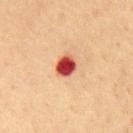Clinical summary: From the chest. A lesion tile, about 15 mm wide, cut from a 3D total-body photograph. A male patient in their 60s.